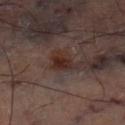Case summary:
* biopsy status · catalogued during a skin exam; not biopsied
* size · ~3 mm (longest diameter)
* site · the left thigh
* TBP lesion metrics · a border-irregularity rating of about 3/10, a color-variation rating of about 4/10, and radial color variation of about 1; a classifier nevus-likeness of about 75/100 and a detector confidence of about 100 out of 100 that the crop contains a lesion
* imaging modality · ~15 mm crop, total-body skin-cancer survey
* illumination · cross-polarized